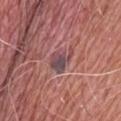notes: no biopsy performed (imaged during a skin exam) | lesion diameter: about 3.5 mm | location: the upper back | image-analysis metrics: a lesion–skin lightness drop of about 9; an automated nevus-likeness rating near 5 out of 100 | subject: male, aged around 60 | image source: ~15 mm crop, total-body skin-cancer survey | lighting: white-light.